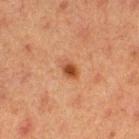Part of a total-body skin-imaging series; this lesion was reviewed on a skin check and was not flagged for biopsy. A 15 mm close-up tile from a total-body photography series done for melanoma screening. The patient is a female aged 38 to 42. Located on the right thigh.